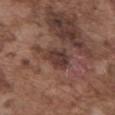No biopsy was performed on this lesion — it was imaged during a full skin examination and was not determined to be concerning. An algorithmic analysis of the crop reported a lesion color around L≈37 a*≈18 b*≈21 in CIELAB, roughly 10 lightness units darker than nearby skin, and a lesion-to-skin contrast of about 8.5 (normalized; higher = more distinct). It also reported a border-irregularity rating of about 3/10, a within-lesion color-variation index near 3.5/10, and peripheral color asymmetry of about 1. It also reported an automated nevus-likeness rating near 15 out of 100. Located on the abdomen. A lesion tile, about 15 mm wide, cut from a 3D total-body photograph. The subject is a male roughly 75 years of age. Longest diameter approximately 3.5 mm. The tile uses white-light illumination.